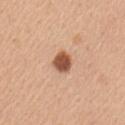The lesion was tiled from a total-body skin photograph and was not biopsied. Approximately 2.5 mm at its widest. A region of skin cropped from a whole-body photographic capture, roughly 15 mm wide. On the left upper arm. The lesion-visualizer software estimated a footprint of about 5 mm², a shape eccentricity near 0.45, and two-axis asymmetry of about 0.2. The software also gave a nevus-likeness score of about 100/100. A female patient, aged 43 to 47.